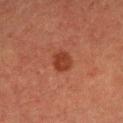The lesion was photographed on a routine skin check and not biopsied; there is no pathology result.